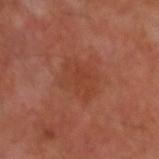workup: imaged on a skin check; not biopsied | anatomic site: the left arm | automated lesion analysis: an area of roughly 12 mm² and a symmetry-axis asymmetry near 0.3; roughly 5 lightness units darker than nearby skin; a peripheral color-asymmetry measure near 1; a nevus-likeness score of about 0/100 and lesion-presence confidence of about 100/100 | imaging modality: total-body-photography crop, ~15 mm field of view | subject: male, aged approximately 50.